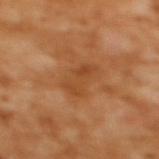The lesion is on the back. This is a cross-polarized tile. A roughly 15 mm field-of-view crop from a total-body skin photograph. A female patient, roughly 55 years of age. Measured at roughly 4.5 mm in maximum diameter.